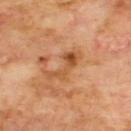Q: Is there a histopathology result?
A: no biopsy performed (imaged during a skin exam)
Q: Who is the patient?
A: male, aged approximately 70
Q: Illumination type?
A: cross-polarized illumination
Q: Where on the body is the lesion?
A: the upper back
Q: Automated lesion metrics?
A: a lesion color around L≈52 a*≈25 b*≈40 in CIELAB, about 9 CIELAB-L* units darker than the surrounding skin, and a normalized border contrast of about 7; a nevus-likeness score of about 0/100
Q: What is the imaging modality?
A: total-body-photography crop, ~15 mm field of view